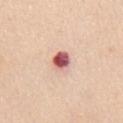{"site": "chest", "patient": {"sex": "female", "age_approx": 55}, "lighting": "white-light", "automated_metrics": {"area_mm2_approx": 4.5, "shape_asymmetry": 0.15, "border_irregularity_0_10": 1.5, "peripheral_color_asymmetry": 1.0}, "lesion_size": {"long_diameter_mm_approx": 2.5}, "image": {"source": "total-body photography crop", "field_of_view_mm": 15}}